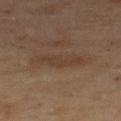notes=total-body-photography surveillance lesion; no biopsy | subject=female, aged 58–62 | site=the upper back | automated lesion analysis=a border-irregularity index near 4.5/10 and radial color variation of about 0.5; a classifier nevus-likeness of about 0/100 and a detector confidence of about 100 out of 100 that the crop contains a lesion | illumination=cross-polarized illumination | size=~4.5 mm (longest diameter) | acquisition=total-body-photography crop, ~15 mm field of view.